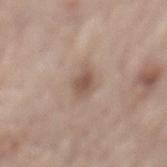Part of a total-body skin-imaging series; this lesion was reviewed on a skin check and was not flagged for biopsy.
From the mid back.
A region of skin cropped from a whole-body photographic capture, roughly 15 mm wide.
A male patient, aged 53–57.
This is a white-light tile.